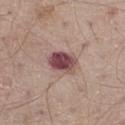Q: Was this lesion biopsied?
A: total-body-photography surveillance lesion; no biopsy
Q: Lesion size?
A: about 3.5 mm
Q: Where on the body is the lesion?
A: the left thigh
Q: What are the patient's age and sex?
A: male, aged around 65
Q: What did automated image analysis measure?
A: a footprint of about 8 mm², an outline eccentricity of about 0.7 (0 = round, 1 = elongated), and two-axis asymmetry of about 0.15; a border-irregularity rating of about 2/10, internal color variation of about 6 on a 0–10 scale, and a peripheral color-asymmetry measure near 1.5; an automated nevus-likeness rating near 5 out of 100 and a lesion-detection confidence of about 100/100
Q: What kind of image is this?
A: total-body-photography crop, ~15 mm field of view
Q: Illumination type?
A: white-light